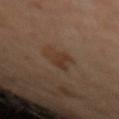No biopsy was performed on this lesion — it was imaged during a full skin examination and was not determined to be concerning.
The subject is a female aged around 60.
A 15 mm close-up tile from a total-body photography series done for melanoma screening.
Located on the arm.